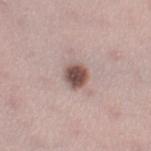Assessment: Captured during whole-body skin photography for melanoma surveillance; the lesion was not biopsied. Clinical summary: Measured at roughly 3 mm in maximum diameter. A 15 mm crop from a total-body photograph taken for skin-cancer surveillance. The lesion is located on the leg. The tile uses white-light illumination. A female subject in their mid- to late 30s. The total-body-photography lesion software estimated a mean CIELAB color near L≈50 a*≈18 b*≈21 and a normalized lesion–skin contrast near 11.5. And it measured border irregularity of about 1.5 on a 0–10 scale, a within-lesion color-variation index near 3.5/10, and peripheral color asymmetry of about 1.